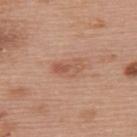  biopsy_status: not biopsied; imaged during a skin examination
  automated_metrics:
    area_mm2_approx: 6.5
    eccentricity: 0.85
    shape_asymmetry: 0.3
    border_irregularity_0_10: 2.5
    color_variation_0_10: 5.5
  site: back
  patient:
    sex: female
    age_approx: 60
  lighting: white-light
  image:
    source: total-body photography crop
    field_of_view_mm: 15